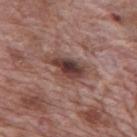workup: total-body-photography surveillance lesion; no biopsy
tile lighting: white-light illumination
acquisition: ~15 mm tile from a whole-body skin photo
subject: male, aged around 70
automated metrics: a lesion area of about 8.5 mm², a shape eccentricity near 0.85, and a symmetry-axis asymmetry near 0.25; a border-irregularity rating of about 3.5/10, a color-variation rating of about 7.5/10, and peripheral color asymmetry of about 2; a classifier nevus-likeness of about 10/100 and a detector confidence of about 100 out of 100 that the crop contains a lesion
lesion diameter: about 5 mm
anatomic site: the mid back